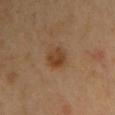Captured during whole-body skin photography for melanoma surveillance; the lesion was not biopsied.
A female subject, aged 48 to 52.
Located on the chest.
A 15 mm crop from a total-body photograph taken for skin-cancer surveillance.